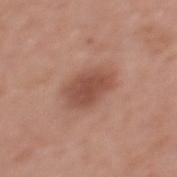A lesion tile, about 15 mm wide, cut from a 3D total-body photograph.
The subject is a female about 35 years old.
The lesion is located on the upper back.
This is a white-light tile.